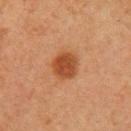This is a cross-polarized tile.
A 15 mm close-up extracted from a 3D total-body photography capture.
The recorded lesion diameter is about 3.5 mm.
From the left upper arm.
An algorithmic analysis of the crop reported an automated nevus-likeness rating near 100 out of 100 and a lesion-detection confidence of about 100/100.
A female subject aged 58 to 62.